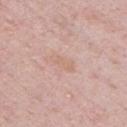workup: catalogued during a skin exam; not biopsied | illumination: white-light illumination | image source: total-body-photography crop, ~15 mm field of view | site: the chest | patient: male, aged 48–52 | diameter: about 3.5 mm | automated lesion analysis: a border-irregularity rating of about 2.5/10, a within-lesion color-variation index near 1/10, and a peripheral color-asymmetry measure near 0; a classifier nevus-likeness of about 0/100 and a lesion-detection confidence of about 100/100.